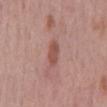  biopsy_status: not biopsied; imaged during a skin examination
  site: back
  patient:
    sex: male
    age_approx: 60
  image:
    source: total-body photography crop
    field_of_view_mm: 15